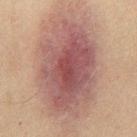biopsy status = imaged on a skin check; not biopsied
subject = male, in their mid- to late 40s
anatomic site = the abdomen
image source = 15 mm crop, total-body photography